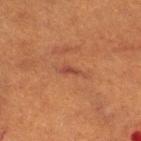{
  "biopsy_status": "not biopsied; imaged during a skin examination",
  "image": {
    "source": "total-body photography crop",
    "field_of_view_mm": 15
  },
  "site": "right thigh",
  "patient": {
    "sex": "female",
    "age_approx": 55
  },
  "lighting": "cross-polarized",
  "lesion_size": {
    "long_diameter_mm_approx": 3.0
  },
  "automated_metrics": {
    "area_mm2_approx": 2.5,
    "eccentricity": 0.95,
    "shape_asymmetry": 0.35,
    "border_irregularity_0_10": 4.5,
    "color_variation_0_10": 0.0,
    "peripheral_color_asymmetry": 0.0,
    "nevus_likeness_0_100": 0,
    "lesion_detection_confidence_0_100": 90
  }
}